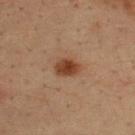workup: catalogued during a skin exam; not biopsied
anatomic site: the back
subject: male, about 35 years old
imaging modality: total-body-photography crop, ~15 mm field of view